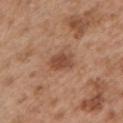Q: Was a biopsy performed?
A: catalogued during a skin exam; not biopsied
Q: Illumination type?
A: white-light illumination
Q: Who is the patient?
A: male, aged 53 to 57
Q: What is the anatomic site?
A: the left upper arm
Q: What kind of image is this?
A: 15 mm crop, total-body photography